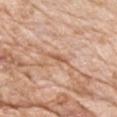Notes:
– follow-up: total-body-photography surveillance lesion; no biopsy
– image source: ~15 mm crop, total-body skin-cancer survey
– site: the chest
– lighting: white-light
– subject: male, in their mid- to late 70s
– lesion size: ≈2.5 mm